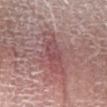Q: Was a biopsy performed?
A: total-body-photography surveillance lesion; no biopsy
Q: How was the tile lit?
A: white-light illumination
Q: Who is the patient?
A: female, about 70 years old
Q: What is the anatomic site?
A: the left lower leg
Q: Automated lesion metrics?
A: internal color variation of about 5.5 on a 0–10 scale and radial color variation of about 2
Q: Lesion size?
A: ~5.5 mm (longest diameter)
Q: What is the imaging modality?
A: 15 mm crop, total-body photography